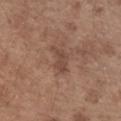This lesion was catalogued during total-body skin photography and was not selected for biopsy.
On the left forearm.
A region of skin cropped from a whole-body photographic capture, roughly 15 mm wide.
A male subject approximately 70 years of age.